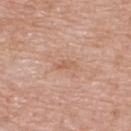This lesion was catalogued during total-body skin photography and was not selected for biopsy.
The total-body-photography lesion software estimated a lesion area of about 2.5 mm², an eccentricity of roughly 0.9, and a shape-asymmetry score of about 0.35 (0 = symmetric). And it measured a mean CIELAB color near L≈60 a*≈21 b*≈32 and a normalized lesion–skin contrast near 5.5. The software also gave border irregularity of about 4 on a 0–10 scale, a color-variation rating of about 0/10, and radial color variation of about 0. The analysis additionally found an automated nevus-likeness rating near 0 out of 100 and lesion-presence confidence of about 100/100.
This is a white-light tile.
The patient is a female about 65 years old.
A 15 mm crop from a total-body photograph taken for skin-cancer surveillance.
Measured at roughly 2.5 mm in maximum diameter.
Located on the back.